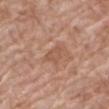<lesion>
<biopsy_status>not biopsied; imaged during a skin examination</biopsy_status>
</lesion>